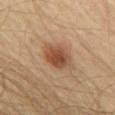workup = total-body-photography surveillance lesion; no biopsy
anatomic site = the chest
image source = total-body-photography crop, ~15 mm field of view
subject = male, in their mid- to late 60s
size = ~3.5 mm (longest diameter)
lighting = cross-polarized